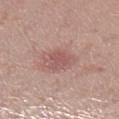The lesion was photographed on a routine skin check and not biopsied; there is no pathology result. Cropped from a total-body skin-imaging series; the visible field is about 15 mm. The lesion is located on the leg. A female patient, aged approximately 30.